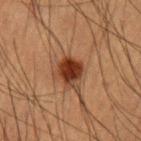This lesion was catalogued during total-body skin photography and was not selected for biopsy.
The subject is a male aged around 50.
The recorded lesion diameter is about 3.5 mm.
From the left upper arm.
A 15 mm crop from a total-body photograph taken for skin-cancer surveillance.
This is a cross-polarized tile.